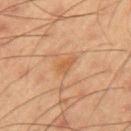Q: Was a biopsy performed?
A: catalogued during a skin exam; not biopsied
Q: What lighting was used for the tile?
A: cross-polarized illumination
Q: What is the lesion's diameter?
A: about 2.5 mm
Q: What is the anatomic site?
A: the arm
Q: Patient demographics?
A: male, aged 53–57
Q: How was this image acquired?
A: total-body-photography crop, ~15 mm field of view